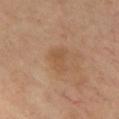| feature | finding |
|---|---|
| workup | catalogued during a skin exam; not biopsied |
| body site | the chest |
| size | ~3 mm (longest diameter) |
| illumination | cross-polarized illumination |
| imaging modality | ~15 mm tile from a whole-body skin photo |
| subject | male, aged 53–57 |